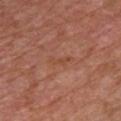Case summary:
* workup — imaged on a skin check; not biopsied
* patient — male, roughly 75 years of age
* lesion diameter — about 3 mm
* illumination — white-light illumination
* body site — the front of the torso
* imaging modality — ~15 mm tile from a whole-body skin photo